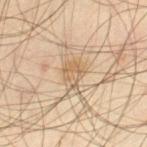Assessment:
Imaged during a routine full-body skin examination; the lesion was not biopsied and no histopathology is available.
Image and clinical context:
Approximately 3 mm at its widest. A male subject, aged 38 to 42. The tile uses cross-polarized illumination. Cropped from a total-body skin-imaging series; the visible field is about 15 mm. From the left thigh. An algorithmic analysis of the crop reported an outline eccentricity of about 0.55 (0 = round, 1 = elongated) and a shape-asymmetry score of about 0.3 (0 = symmetric). It also reported about 8 CIELAB-L* units darker than the surrounding skin and a normalized border contrast of about 6. It also reported peripheral color asymmetry of about 1.5. The analysis additionally found a nevus-likeness score of about 0/100 and a lesion-detection confidence of about 85/100.